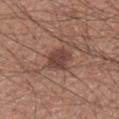– workup: imaged on a skin check; not biopsied
– patient: male, in their mid-50s
– acquisition: ~15 mm tile from a whole-body skin photo